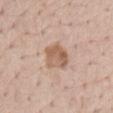Clinical impression:
The lesion was tiled from a total-body skin photograph and was not biopsied.
Image and clinical context:
The lesion's longest dimension is about 3.5 mm. A female patient, in their mid-70s. Cropped from a total-body skin-imaging series; the visible field is about 15 mm. The lesion is located on the lower back. The total-body-photography lesion software estimated an area of roughly 8.5 mm² and two-axis asymmetry of about 0.25. And it measured a lesion color around L≈59 a*≈19 b*≈30 in CIELAB, about 11 CIELAB-L* units darker than the surrounding skin, and a lesion-to-skin contrast of about 7.5 (normalized; higher = more distinct).